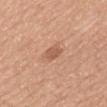<tbp_lesion>
<biopsy_status>not biopsied; imaged during a skin examination</biopsy_status>
<lesion_size>
  <long_diameter_mm_approx>2.5</long_diameter_mm_approx>
</lesion_size>
<patient>
  <sex>female</sex>
  <age_approx>55</age_approx>
</patient>
<site>mid back</site>
<image>
  <source>total-body photography crop</source>
  <field_of_view_mm>15</field_of_view_mm>
</image>
</tbp_lesion>